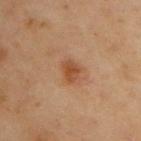Captured during whole-body skin photography for melanoma surveillance; the lesion was not biopsied. The patient is a male in their mid- to late 50s. Longest diameter approximately 2.5 mm. The lesion-visualizer software estimated a mean CIELAB color near L≈39 a*≈20 b*≈30 and a lesion–skin lightness drop of about 8. It also reported a border-irregularity index near 2.5/10, a color-variation rating of about 2/10, and a peripheral color-asymmetry measure near 0.5. The software also gave an automated nevus-likeness rating near 85 out of 100 and lesion-presence confidence of about 100/100. A lesion tile, about 15 mm wide, cut from a 3D total-body photograph. The lesion is located on the chest.